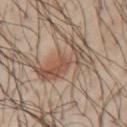No biopsy was performed on this lesion — it was imaged during a full skin examination and was not determined to be concerning. A male patient, aged 38 to 42. Located on the arm. Captured under cross-polarized illumination. A region of skin cropped from a whole-body photographic capture, roughly 15 mm wide. Measured at roughly 6 mm in maximum diameter.